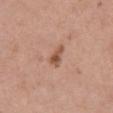Q: Was this lesion biopsied?
A: imaged on a skin check; not biopsied
Q: Patient demographics?
A: female, aged 58–62
Q: What lighting was used for the tile?
A: white-light illumination
Q: What did automated image analysis measure?
A: a lesion area of about 3.5 mm² and an outline eccentricity of about 0.85 (0 = round, 1 = elongated); an average lesion color of about L≈53 a*≈22 b*≈30 (CIELAB) and about 11 CIELAB-L* units darker than the surrounding skin; a border-irregularity rating of about 4/10, a color-variation rating of about 3/10, and peripheral color asymmetry of about 1; a nevus-likeness score of about 65/100 and lesion-presence confidence of about 100/100
Q: Lesion location?
A: the abdomen
Q: What is the lesion's diameter?
A: about 3 mm
Q: What is the imaging modality?
A: ~15 mm crop, total-body skin-cancer survey